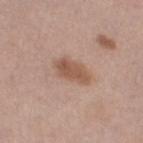This lesion was catalogued during total-body skin photography and was not selected for biopsy.
A female subject aged 53 to 57.
A 15 mm crop from a total-body photograph taken for skin-cancer surveillance.
From the left thigh.